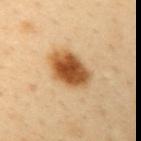Recorded during total-body skin imaging; not selected for excision or biopsy.
Imaged with cross-polarized lighting.
The lesion is located on the left upper arm.
The total-body-photography lesion software estimated an area of roughly 14 mm², an outline eccentricity of about 0.75 (0 = round, 1 = elongated), and a shape-asymmetry score of about 0.15 (0 = symmetric). And it measured border irregularity of about 1.5 on a 0–10 scale and internal color variation of about 6 on a 0–10 scale.
The recorded lesion diameter is about 5 mm.
A male patient about 40 years old.
A 15 mm close-up tile from a total-body photography series done for melanoma screening.